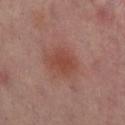Q: Is there a histopathology result?
A: catalogued during a skin exam; not biopsied
Q: What did automated image analysis measure?
A: a lesion color around L≈45 a*≈24 b*≈26 in CIELAB and a lesion-to-skin contrast of about 6.5 (normalized; higher = more distinct); an automated nevus-likeness rating near 30 out of 100 and a detector confidence of about 100 out of 100 that the crop contains a lesion
Q: Lesion size?
A: ≈4 mm
Q: How was this image acquired?
A: 15 mm crop, total-body photography
Q: What lighting was used for the tile?
A: cross-polarized
Q: What are the patient's age and sex?
A: female, aged 38 to 42
Q: Lesion location?
A: the right lower leg